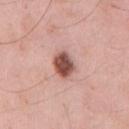{
  "site": "front of the torso",
  "image": {
    "source": "total-body photography crop",
    "field_of_view_mm": 15
  },
  "lesion_size": {
    "long_diameter_mm_approx": 3.0
  },
  "patient": {
    "sex": "male",
    "age_approx": 60
  }
}